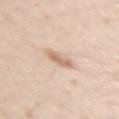Assessment:
The lesion was tiled from a total-body skin photograph and was not biopsied.
Acquisition and patient details:
A female subject, aged 23–27. A 15 mm close-up extracted from a 3D total-body photography capture. The lesion is on the arm.